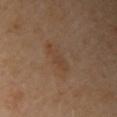The lesion was photographed on a routine skin check and not biopsied; there is no pathology result. The lesion is located on the right upper arm. A 15 mm close-up extracted from a 3D total-body photography capture. A female subject, aged 63 to 67.